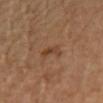Impression:
Imaged during a routine full-body skin examination; the lesion was not biopsied and no histopathology is available.
Background:
A close-up tile cropped from a whole-body skin photograph, about 15 mm across. On the right upper arm. The patient is aged 58 to 62. Automated image analysis of the tile measured a lesion color around L≈41 a*≈20 b*≈31 in CIELAB and roughly 7 lightness units darker than nearby skin. The software also gave a border-irregularity index near 4/10, a within-lesion color-variation index near 3/10, and a peripheral color-asymmetry measure near 1. The recorded lesion diameter is about 3 mm.